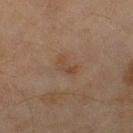The lesion was tiled from a total-body skin photograph and was not biopsied. This is a cross-polarized tile. A roughly 15 mm field-of-view crop from a total-body skin photograph. Measured at roughly 2.5 mm in maximum diameter. From the right lower leg. A female subject, roughly 60 years of age. The total-body-photography lesion software estimated an outline eccentricity of about 0.85 (0 = round, 1 = elongated) and a symmetry-axis asymmetry near 0.35. The analysis additionally found a mean CIELAB color near L≈40 a*≈15 b*≈26 and a normalized lesion–skin contrast near 5. It also reported a color-variation rating of about 1/10 and radial color variation of about 0. The analysis additionally found an automated nevus-likeness rating near 0 out of 100.